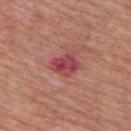The subject is a male aged 63–67. On the upper back. Automated image analysis of the tile measured a within-lesion color-variation index near 5.5/10. The analysis additionally found a classifier nevus-likeness of about 0/100 and a lesion-detection confidence of about 100/100. Imaged with white-light lighting. A roughly 15 mm field-of-view crop from a total-body skin photograph.